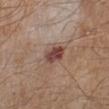size: ≈3 mm
image source: ~15 mm crop, total-body skin-cancer survey
subject: male, roughly 65 years of age
body site: the leg
illumination: white-light illumination
TBP lesion metrics: a within-lesion color-variation index near 4/10